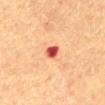{"biopsy_status": "not biopsied; imaged during a skin examination", "patient": {"sex": "male", "age_approx": 60}, "lesion_size": {"long_diameter_mm_approx": 2.5}, "image": {"source": "total-body photography crop", "field_of_view_mm": 15}, "lighting": "cross-polarized", "site": "mid back"}